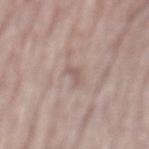Part of a total-body skin-imaging series; this lesion was reviewed on a skin check and was not flagged for biopsy. The recorded lesion diameter is about 2.5 mm. The subject is a male aged 63 to 67. The lesion is located on the back. This is a white-light tile. An algorithmic analysis of the crop reported an eccentricity of roughly 0.9 and a shape-asymmetry score of about 0.45 (0 = symmetric). It also reported a lesion color around L≈56 a*≈16 b*≈21 in CIELAB, a lesion–skin lightness drop of about 7, and a lesion-to-skin contrast of about 5 (normalized; higher = more distinct). And it measured a within-lesion color-variation index near 0/10 and a peripheral color-asymmetry measure near 0. A 15 mm close-up extracted from a 3D total-body photography capture.